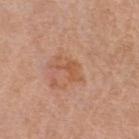A region of skin cropped from a whole-body photographic capture, roughly 15 mm wide. Imaged with white-light lighting. A female subject roughly 65 years of age. On the left lower leg.